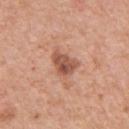Q: Was a biopsy performed?
A: imaged on a skin check; not biopsied
Q: What is the lesion's diameter?
A: ~3.5 mm (longest diameter)
Q: Who is the patient?
A: female, roughly 40 years of age
Q: How was this image acquired?
A: ~15 mm tile from a whole-body skin photo
Q: Where on the body is the lesion?
A: the arm